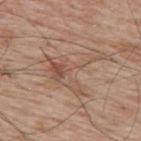This lesion was catalogued during total-body skin photography and was not selected for biopsy.
The lesion's longest dimension is about 7.5 mm.
On the upper back.
A 15 mm close-up tile from a total-body photography series done for melanoma screening.
The subject is a male aged 68 to 72.